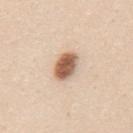Clinical impression: Recorded during total-body skin imaging; not selected for excision or biopsy. Image and clinical context: About 4 mm across. A male patient, approximately 25 years of age. This image is a 15 mm lesion crop taken from a total-body photograph. Captured under white-light illumination. The lesion is on the mid back.